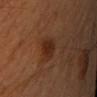Impression:
The lesion was tiled from a total-body skin photograph and was not biopsied.
Background:
A male subject, aged around 50. Measured at roughly 3 mm in maximum diameter. The lesion is on the left upper arm. This is a cross-polarized tile. Cropped from a total-body skin-imaging series; the visible field is about 15 mm.